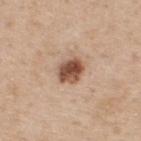Recorded during total-body skin imaging; not selected for excision or biopsy. The patient is a male aged approximately 60. On the upper back. Cropped from a total-body skin-imaging series; the visible field is about 15 mm. The tile uses white-light illumination.